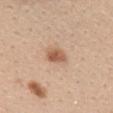biopsy status — no biopsy performed (imaged during a skin exam) | patient — female, aged 23 to 27 | anatomic site — the upper back | acquisition — 15 mm crop, total-body photography.